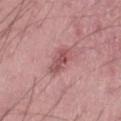Assessment:
The lesion was tiled from a total-body skin photograph and was not biopsied.